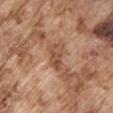Clinical summary:
From the right upper arm. Automated image analysis of the tile measured lesion-presence confidence of about 65/100. About 3.5 mm across. A roughly 15 mm field-of-view crop from a total-body skin photograph. A male patient, aged around 75. This is a white-light tile.